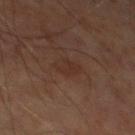{"site": "leg", "patient": {"sex": "male", "age_approx": 60}, "lesion_size": {"long_diameter_mm_approx": 2.5}, "image": {"source": "total-body photography crop", "field_of_view_mm": 15}, "lighting": "cross-polarized"}